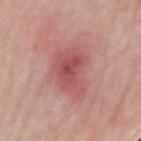biopsy status = catalogued during a skin exam; not biopsied | imaging modality = ~15 mm tile from a whole-body skin photo | TBP lesion metrics = an average lesion color of about L≈54 a*≈28 b*≈23 (CIELAB) and roughly 10 lightness units darker than nearby skin; border irregularity of about 3 on a 0–10 scale and radial color variation of about 1; a classifier nevus-likeness of about 0/100 and a lesion-detection confidence of about 100/100 | illumination = white-light | lesion size = ≈6.5 mm | subject = male, aged around 75 | location = the right forearm.